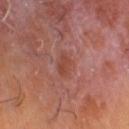Clinical impression:
Captured during whole-body skin photography for melanoma surveillance; the lesion was not biopsied.
Image and clinical context:
A roughly 15 mm field-of-view crop from a total-body skin photograph. On the left lower leg. A male subject, aged 58 to 62.